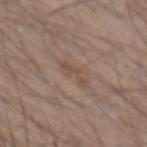Clinical impression:
Captured during whole-body skin photography for melanoma surveillance; the lesion was not biopsied.
Background:
A male subject roughly 50 years of age. The lesion's longest dimension is about 3.5 mm. This is a white-light tile. The total-body-photography lesion software estimated an average lesion color of about L≈49 a*≈15 b*≈26 (CIELAB), about 6 CIELAB-L* units darker than the surrounding skin, and a normalized border contrast of about 5.5. The software also gave a border-irregularity rating of about 6.5/10 and a peripheral color-asymmetry measure near 0. The analysis additionally found a classifier nevus-likeness of about 0/100 and lesion-presence confidence of about 100/100. A region of skin cropped from a whole-body photographic capture, roughly 15 mm wide. From the left forearm.